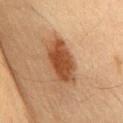biopsy status=total-body-photography surveillance lesion; no biopsy
illumination=cross-polarized
lesion diameter=~6 mm (longest diameter)
patient=male, aged approximately 65
body site=the abdomen
image=total-body-photography crop, ~15 mm field of view